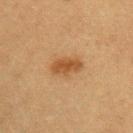Part of a total-body skin-imaging series; this lesion was reviewed on a skin check and was not flagged for biopsy. Measured at roughly 4 mm in maximum diameter. A region of skin cropped from a whole-body photographic capture, roughly 15 mm wide. This is a cross-polarized tile. An algorithmic analysis of the crop reported a normalized border contrast of about 8. A male subject roughly 40 years of age. On the left upper arm.